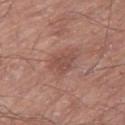• notes: total-body-photography surveillance lesion; no biopsy
• diameter: ≈3.5 mm
• location: the leg
• automated metrics: a lesion color around L≈49 a*≈22 b*≈25 in CIELAB, roughly 8 lightness units darker than nearby skin, and a lesion-to-skin contrast of about 6 (normalized; higher = more distinct); lesion-presence confidence of about 100/100
• image source: total-body-photography crop, ~15 mm field of view
• patient: male, aged 68 to 72
• lighting: white-light